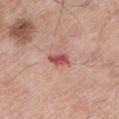This lesion was catalogued during total-body skin photography and was not selected for biopsy.
Cropped from a whole-body photographic skin survey; the tile spans about 15 mm.
Located on the left lower leg.
The patient is a male approximately 80 years of age.